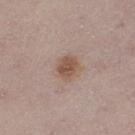A region of skin cropped from a whole-body photographic capture, roughly 15 mm wide.
A female patient, aged around 25.
Automated tile analysis of the lesion measured a footprint of about 6 mm² and a shape-asymmetry score of about 0.2 (0 = symmetric). It also reported a mean CIELAB color near L≈52 a*≈18 b*≈27, about 10 CIELAB-L* units darker than the surrounding skin, and a lesion-to-skin contrast of about 8.5 (normalized; higher = more distinct). The analysis additionally found peripheral color asymmetry of about 1.
The lesion is located on the left thigh.
The lesion's longest dimension is about 3 mm.
Captured under white-light illumination.